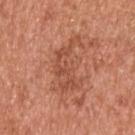Case summary:
- anatomic site · the upper back
- imaging modality · 15 mm crop, total-body photography
- illumination · white-light illumination
- subject · male, in their mid-50s